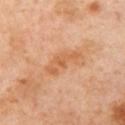follow-up: no biopsy performed (imaged during a skin exam) | illumination: cross-polarized | image: total-body-photography crop, ~15 mm field of view | diameter: ≈4.5 mm | location: the left upper arm | subject: female, roughly 40 years of age | TBP lesion metrics: a footprint of about 7.5 mm², an outline eccentricity of about 0.9 (0 = round, 1 = elongated), and a shape-asymmetry score of about 0.4 (0 = symmetric); a nevus-likeness score of about 0/100.